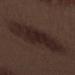Part of a total-body skin-imaging series; this lesion was reviewed on a skin check and was not flagged for biopsy.
Approximately 7 mm at its widest.
A 15 mm close-up extracted from a 3D total-body photography capture.
From the left forearm.
Captured under white-light illumination.
A male subject, roughly 70 years of age.